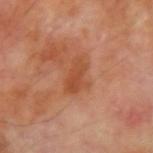lesion size: ≈4 mm | image source: total-body-photography crop, ~15 mm field of view | TBP lesion metrics: an area of roughly 6.5 mm², an eccentricity of roughly 0.85, and a symmetry-axis asymmetry near 0.3; a within-lesion color-variation index near 2.5/10 and radial color variation of about 1 | tile lighting: cross-polarized illumination | patient: male, roughly 70 years of age | anatomic site: the right upper arm.